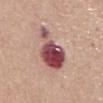{
  "biopsy_status": "not biopsied; imaged during a skin examination",
  "site": "abdomen",
  "patient": {
    "sex": "female",
    "age_approx": 55
  },
  "lesion_size": {
    "long_diameter_mm_approx": 6.5
  },
  "image": {
    "source": "total-body photography crop",
    "field_of_view_mm": 15
  }
}